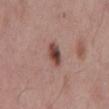Impression: The lesion was photographed on a routine skin check and not biopsied; there is no pathology result. Acquisition and patient details: The total-body-photography lesion software estimated a footprint of about 5 mm², a shape eccentricity near 0.8, and a symmetry-axis asymmetry near 0.15. The software also gave a lesion–skin lightness drop of about 15 and a normalized border contrast of about 10.5. It also reported a classifier nevus-likeness of about 95/100 and lesion-presence confidence of about 100/100. A lesion tile, about 15 mm wide, cut from a 3D total-body photograph. On the mid back. The lesion's longest dimension is about 3 mm. A male subject aged around 55.